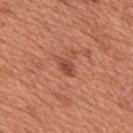Q: Was a biopsy performed?
A: no biopsy performed (imaged during a skin exam)
Q: What is the imaging modality?
A: ~15 mm crop, total-body skin-cancer survey
Q: Automated lesion metrics?
A: border irregularity of about 2.5 on a 0–10 scale and radial color variation of about 0.5; a nevus-likeness score of about 15/100 and lesion-presence confidence of about 100/100
Q: How was the tile lit?
A: white-light illumination
Q: Where on the body is the lesion?
A: the upper back
Q: What is the lesion's diameter?
A: ~2.5 mm (longest diameter)
Q: Patient demographics?
A: male, aged approximately 65